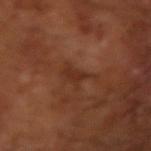Imaged during a routine full-body skin examination; the lesion was not biopsied and no histopathology is available.
A male subject, aged approximately 65.
Measured at roughly 4 mm in maximum diameter.
Automated tile analysis of the lesion measured a nevus-likeness score of about 0/100.
Imaged with cross-polarized lighting.
A 15 mm close-up extracted from a 3D total-body photography capture.